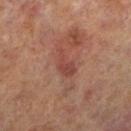biopsy status: total-body-photography surveillance lesion; no biopsy
size: ≈4 mm
location: the left lower leg
patient: male, in their 70s
acquisition: ~15 mm tile from a whole-body skin photo
illumination: cross-polarized